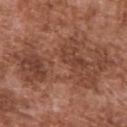The lesion was photographed on a routine skin check and not biopsied; there is no pathology result. From the upper back. The patient is a male aged 73–77. Longest diameter approximately 14.5 mm. A close-up tile cropped from a whole-body skin photograph, about 15 mm across.